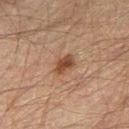No biopsy was performed on this lesion — it was imaged during a full skin examination and was not determined to be concerning.